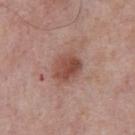Part of a total-body skin-imaging series; this lesion was reviewed on a skin check and was not flagged for biopsy.
Approximately 4 mm at its widest.
This is a white-light tile.
The lesion-visualizer software estimated an area of roughly 9.5 mm², an outline eccentricity of about 0.55 (0 = round, 1 = elongated), and a shape-asymmetry score of about 0.15 (0 = symmetric). The software also gave a color-variation rating of about 5/10.
A 15 mm close-up tile from a total-body photography series done for melanoma screening.
The lesion is located on the front of the torso.
The patient is a male about 75 years old.